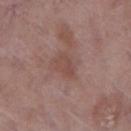The subject is a female aged around 70. The tile uses white-light illumination. From the right lower leg. A 15 mm crop from a total-body photograph taken for skin-cancer surveillance. Approximately 3 mm at its widest.